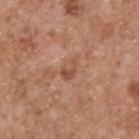anatomic site: the upper back
subject: male, in their mid-50s
automated lesion analysis: roughly 8 lightness units darker than nearby skin and a normalized lesion–skin contrast near 6; internal color variation of about 4.5 on a 0–10 scale; a nevus-likeness score of about 0/100 and a detector confidence of about 100 out of 100 that the crop contains a lesion
lighting: white-light illumination
image source: ~15 mm tile from a whole-body skin photo